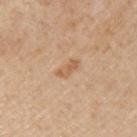This lesion was catalogued during total-body skin photography and was not selected for biopsy.
The total-body-photography lesion software estimated an average lesion color of about L≈60 a*≈19 b*≈34 (CIELAB), a lesion–skin lightness drop of about 8, and a lesion-to-skin contrast of about 6 (normalized; higher = more distinct). It also reported a border-irregularity index near 3.5/10, a within-lesion color-variation index near 2.5/10, and a peripheral color-asymmetry measure near 1.
Approximately 3 mm at its widest.
A male patient aged around 55.
The lesion is on the left upper arm.
Cropped from a total-body skin-imaging series; the visible field is about 15 mm.
Imaged with white-light lighting.